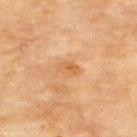Findings:
– biopsy status · imaged on a skin check; not biopsied
– image · ~15 mm tile from a whole-body skin photo
– size · ~2.5 mm (longest diameter)
– patient · male, aged approximately 70
– tile lighting · cross-polarized illumination
– body site · the upper back